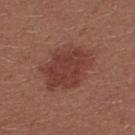Assessment:
Captured during whole-body skin photography for melanoma surveillance; the lesion was not biopsied.
Clinical summary:
A 15 mm crop from a total-body photograph taken for skin-cancer surveillance. A female patient, aged around 25. The recorded lesion diameter is about 6.5 mm. Located on the back. Imaged with white-light lighting.